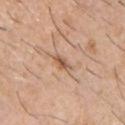Notes:
- notes: imaged on a skin check; not biopsied
- subject: male, roughly 60 years of age
- site: the chest
- acquisition: 15 mm crop, total-body photography